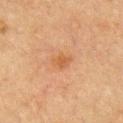Recorded during total-body skin imaging; not selected for excision or biopsy.
A male subject, aged around 65.
Automated image analysis of the tile measured a mean CIELAB color near L≈47 a*≈20 b*≈34. And it measured a nevus-likeness score of about 5/100 and lesion-presence confidence of about 100/100.
The lesion is located on the left upper arm.
Cropped from a whole-body photographic skin survey; the tile spans about 15 mm.
About 2.5 mm across.